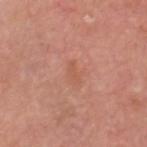Assessment: Part of a total-body skin-imaging series; this lesion was reviewed on a skin check and was not flagged for biopsy. Clinical summary: The tile uses white-light illumination. An algorithmic analysis of the crop reported an area of roughly 2.5 mm², an eccentricity of roughly 0.9, and two-axis asymmetry of about 0.45. And it measured a nevus-likeness score of about 0/100 and a detector confidence of about 100 out of 100 that the crop contains a lesion. Cropped from a whole-body photographic skin survey; the tile spans about 15 mm. A male subject approximately 75 years of age. The lesion is located on the head or neck.